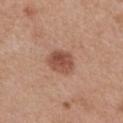{
  "biopsy_status": "not biopsied; imaged during a skin examination",
  "image": {
    "source": "total-body photography crop",
    "field_of_view_mm": 15
  },
  "automated_metrics": {
    "area_mm2_approx": 7.5,
    "eccentricity": 0.6,
    "border_irregularity_0_10": 1.5,
    "color_variation_0_10": 4.0
  },
  "site": "mid back",
  "patient": {
    "sex": "female",
    "age_approx": 40
  },
  "lighting": "white-light"
}